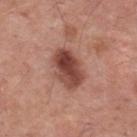Case summary:
* biopsy status · total-body-photography surveillance lesion; no biopsy
* automated metrics · a footprint of about 14 mm², an outline eccentricity of about 0.7 (0 = round, 1 = elongated), and a symmetry-axis asymmetry near 0.15; a classifier nevus-likeness of about 80/100 and lesion-presence confidence of about 100/100
* location · the back
* lighting · white-light
* patient · male, in their mid- to late 50s
* acquisition · 15 mm crop, total-body photography